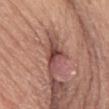This lesion was catalogued during total-body skin photography and was not selected for biopsy.
Approximately 2.5 mm at its widest.
The tile uses white-light illumination.
A 15 mm close-up tile from a total-body photography series done for melanoma screening.
The lesion is located on the leg.
An algorithmic analysis of the crop reported an eccentricity of roughly 0.8 and a shape-asymmetry score of about 0.55 (0 = symmetric). And it measured a lesion color around L≈42 a*≈25 b*≈24 in CIELAB, roughly 15 lightness units darker than nearby skin, and a lesion-to-skin contrast of about 11 (normalized; higher = more distinct). The analysis additionally found border irregularity of about 5.5 on a 0–10 scale, internal color variation of about 0 on a 0–10 scale, and peripheral color asymmetry of about 0.
The patient is a male aged approximately 70.